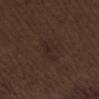The lesion was tiled from a total-body skin photograph and was not biopsied.
Located on the abdomen.
Captured under white-light illumination.
A male patient, aged 68–72.
A close-up tile cropped from a whole-body skin photograph, about 15 mm across.
Approximately 4 mm at its widest.